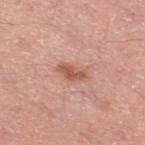No biopsy was performed on this lesion — it was imaged during a full skin examination and was not determined to be concerning.
From the right thigh.
A region of skin cropped from a whole-body photographic capture, roughly 15 mm wide.
A male patient aged around 50.